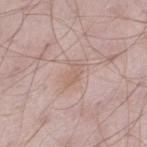- biopsy status: catalogued during a skin exam; not biopsied